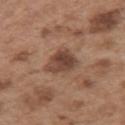Q: Is there a histopathology result?
A: catalogued during a skin exam; not biopsied
Q: What is the lesion's diameter?
A: ~3.5 mm (longest diameter)
Q: What lighting was used for the tile?
A: white-light illumination
Q: Who is the patient?
A: male, aged 53 to 57
Q: Automated lesion metrics?
A: border irregularity of about 2.5 on a 0–10 scale, a color-variation rating of about 5/10, and radial color variation of about 2; an automated nevus-likeness rating near 55 out of 100 and a detector confidence of about 100 out of 100 that the crop contains a lesion
Q: What kind of image is this?
A: total-body-photography crop, ~15 mm field of view
Q: What is the anatomic site?
A: the left upper arm